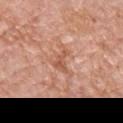biopsy_status: not biopsied; imaged during a skin examination
patient:
  sex: male
  age_approx: 55
lighting: white-light
automated_metrics:
  area_mm2_approx: 4.5
  eccentricity: 0.8
  shape_asymmetry: 0.5
  cielab_L: 57
  cielab_a: 25
  cielab_b: 33
  vs_skin_contrast_norm: 6.5
  border_irregularity_0_10: 5.5
  color_variation_0_10: 2.0
  peripheral_color_asymmetry: 0.5
lesion_size:
  long_diameter_mm_approx: 3.0
image:
  source: total-body photography crop
  field_of_view_mm: 15
site: front of the torso